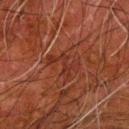| field | value |
|---|---|
| follow-up | no biopsy performed (imaged during a skin exam) |
| body site | the arm |
| image source | total-body-photography crop, ~15 mm field of view |
| patient | male, aged around 80 |
| automated lesion analysis | an average lesion color of about L≈25 a*≈23 b*≈25 (CIELAB), a lesion–skin lightness drop of about 5, and a normalized lesion–skin contrast near 5; border irregularity of about 10 on a 0–10 scale, a within-lesion color-variation index near 0/10, and a peripheral color-asymmetry measure near 0 |